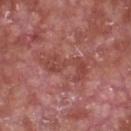No biopsy was performed on this lesion — it was imaged during a full skin examination and was not determined to be concerning. The patient is a male approximately 65 years of age. Cropped from a total-body skin-imaging series; the visible field is about 15 mm. This is a white-light tile. The lesion-visualizer software estimated an automated nevus-likeness rating near 0 out of 100 and lesion-presence confidence of about 80/100. Approximately 6 mm at its widest. On the chest.